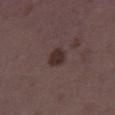No biopsy was performed on this lesion — it was imaged during a full skin examination and was not determined to be concerning. A female patient, in their mid-30s. About 3 mm across. A 15 mm close-up extracted from a 3D total-body photography capture. Located on the leg.